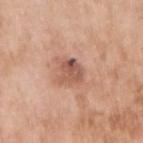The lesion was photographed on a routine skin check and not biopsied; there is no pathology result. The lesion is on the right upper arm. The subject is a female aged around 75. The lesion's longest dimension is about 2.5 mm. A region of skin cropped from a whole-body photographic capture, roughly 15 mm wide.